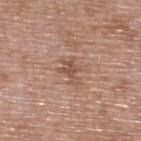Impression: The lesion was tiled from a total-body skin photograph and was not biopsied. Background: A region of skin cropped from a whole-body photographic capture, roughly 15 mm wide. The lesion is on the upper back. A male patient about 50 years old. This is a white-light tile. The total-body-photography lesion software estimated a lesion area of about 4.5 mm², a shape eccentricity near 0.75, and two-axis asymmetry of about 0.55. The software also gave roughly 9 lightness units darker than nearby skin. It also reported a border-irregularity index near 6.5/10, a within-lesion color-variation index near 1.5/10, and a peripheral color-asymmetry measure near 0.5. It also reported a nevus-likeness score of about 0/100 and a detector confidence of about 100 out of 100 that the crop contains a lesion. The recorded lesion diameter is about 3 mm.